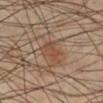Impression:
The lesion was tiled from a total-body skin photograph and was not biopsied.
Image and clinical context:
The recorded lesion diameter is about 3.5 mm. A 15 mm close-up extracted from a 3D total-body photography capture. A male subject roughly 40 years of age. The lesion is on the leg.